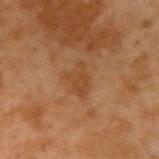Recorded during total-body skin imaging; not selected for excision or biopsy. Located on the right upper arm. Captured under cross-polarized illumination. A 15 mm close-up extracted from a 3D total-body photography capture. Approximately 3.5 mm at its widest. The lesion-visualizer software estimated a footprint of about 6.5 mm², an outline eccentricity of about 0.7 (0 = round, 1 = elongated), and a shape-asymmetry score of about 0.3 (0 = symmetric). A male subject about 45 years old.